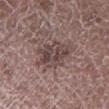Assessment: Captured during whole-body skin photography for melanoma surveillance; the lesion was not biopsied. Context: A 15 mm crop from a total-body photograph taken for skin-cancer surveillance. The recorded lesion diameter is about 6 mm. The lesion is on the leg. The subject is a male aged approximately 75.